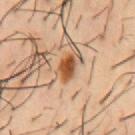This lesion was catalogued during total-body skin photography and was not selected for biopsy.
From the front of the torso.
Cropped from a whole-body photographic skin survey; the tile spans about 15 mm.
A male patient aged 53–57.
The recorded lesion diameter is about 3 mm.